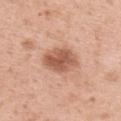Captured during whole-body skin photography for melanoma surveillance; the lesion was not biopsied.
Located on the upper back.
A female patient, aged approximately 50.
A roughly 15 mm field-of-view crop from a total-body skin photograph.
An algorithmic analysis of the crop reported a nevus-likeness score of about 65/100 and a detector confidence of about 100 out of 100 that the crop contains a lesion.